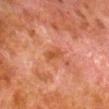Part of a total-body skin-imaging series; this lesion was reviewed on a skin check and was not flagged for biopsy. Imaged with cross-polarized lighting. On the right lower leg. A male patient aged approximately 80. Cropped from a whole-body photographic skin survey; the tile spans about 15 mm. Automated image analysis of the tile measured a mean CIELAB color near L≈40 a*≈24 b*≈32, roughly 6 lightness units darker than nearby skin, and a normalized lesion–skin contrast near 7. The analysis additionally found a border-irregularity rating of about 2.5/10, a within-lesion color-variation index near 0/10, and peripheral color asymmetry of about 0. The recorded lesion diameter is about 2.5 mm.